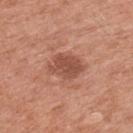Q: Is there a histopathology result?
A: no biopsy performed (imaged during a skin exam)
Q: Where on the body is the lesion?
A: the left upper arm
Q: What are the patient's age and sex?
A: male, aged approximately 55
Q: What kind of image is this?
A: ~15 mm crop, total-body skin-cancer survey
Q: Lesion size?
A: about 4 mm
Q: Illumination type?
A: white-light